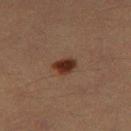biopsy status: imaged on a skin check; not biopsied | image source: 15 mm crop, total-body photography | body site: the left thigh | tile lighting: cross-polarized illumination | lesion size: ≈3 mm | subject: male, aged approximately 40.